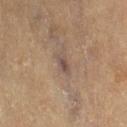Part of a total-body skin-imaging series; this lesion was reviewed on a skin check and was not flagged for biopsy.